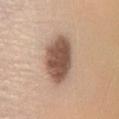Part of a total-body skin-imaging series; this lesion was reviewed on a skin check and was not flagged for biopsy. The subject is a female aged 53–57. A lesion tile, about 15 mm wide, cut from a 3D total-body photograph. The lesion is on the leg.